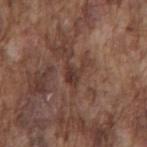Findings:
* patient — male, aged around 75
* tile lighting — white-light
* image — ~15 mm crop, total-body skin-cancer survey
* image-analysis metrics — an area of roughly 4 mm², an eccentricity of roughly 0.6, and a shape-asymmetry score of about 0.5 (0 = symmetric); a mean CIELAB color near L≈36 a*≈19 b*≈23, a lesion–skin lightness drop of about 8, and a normalized lesion–skin contrast near 7.5; border irregularity of about 5 on a 0–10 scale and internal color variation of about 3 on a 0–10 scale
* anatomic site — the mid back